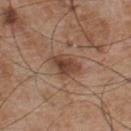| field | value |
|---|---|
| notes | imaged on a skin check; not biopsied |
| patient | male, aged around 55 |
| size | ~3.5 mm (longest diameter) |
| site | the chest |
| imaging modality | 15 mm crop, total-body photography |